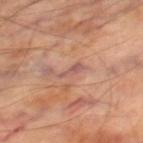notes — no biopsy performed (imaged during a skin exam); patient — male, roughly 70 years of age; body site — the right thigh; image source — 15 mm crop, total-body photography; lesion size — ~3 mm (longest diameter).